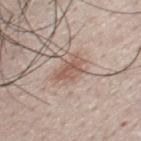| feature | finding |
|---|---|
| notes | total-body-photography surveillance lesion; no biopsy |
| subject | male, in their mid-50s |
| location | the chest |
| size | about 3.5 mm |
| image | ~15 mm crop, total-body skin-cancer survey |